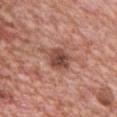- follow-up: no biopsy performed (imaged during a skin exam)
- acquisition: ~15 mm crop, total-body skin-cancer survey
- body site: the chest
- size: about 4.5 mm
- automated metrics: a lesion color around L≈48 a*≈23 b*≈26 in CIELAB; a classifier nevus-likeness of about 45/100 and a detector confidence of about 100 out of 100 that the crop contains a lesion
- patient: male, aged 48 to 52